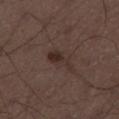<tbp_lesion>
  <biopsy_status>not biopsied; imaged during a skin examination</biopsy_status>
  <patient>
    <sex>male</sex>
    <age_approx>50</age_approx>
  </patient>
  <image>
    <source>total-body photography crop</source>
    <field_of_view_mm>15</field_of_view_mm>
  </image>
  <site>right thigh</site>
</tbp_lesion>